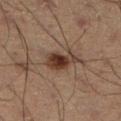notes = imaged on a skin check; not biopsied
tile lighting = cross-polarized
acquisition = 15 mm crop, total-body photography
subject = male, aged approximately 55
site = the leg
diameter = ~4.5 mm (longest diameter)